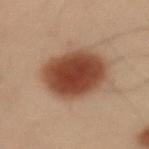follow-up = total-body-photography surveillance lesion; no biopsy
body site = the left upper arm
image = total-body-photography crop, ~15 mm field of view
lighting = cross-polarized
subject = male, approximately 55 years of age